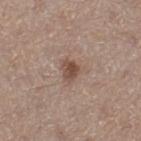No biopsy was performed on this lesion — it was imaged during a full skin examination and was not determined to be concerning. The recorded lesion diameter is about 2.5 mm. Located on the left thigh. The patient is a female roughly 50 years of age. Imaged with white-light lighting. The lesion-visualizer software estimated an outline eccentricity of about 0.6 (0 = round, 1 = elongated) and a symmetry-axis asymmetry near 0.25. Cropped from a whole-body photographic skin survey; the tile spans about 15 mm.